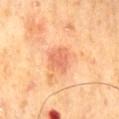Findings:
* follow-up · no biopsy performed (imaged during a skin exam)
* site · the mid back
* illumination · cross-polarized illumination
* subject · male, aged 68–72
* TBP lesion metrics · a footprint of about 6 mm², an eccentricity of roughly 0.55, and a symmetry-axis asymmetry near 0.3; an average lesion color of about L≈54 a*≈25 b*≈33 (CIELAB), a lesion–skin lightness drop of about 8, and a normalized lesion–skin contrast near 6; an automated nevus-likeness rating near 10 out of 100
* lesion diameter · ~3 mm (longest diameter)
* image source · ~15 mm tile from a whole-body skin photo